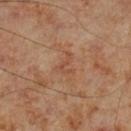| field | value |
|---|---|
| patient | male, in their 70s |
| tile lighting | cross-polarized illumination |
| image | 15 mm crop, total-body photography |
| lesion size | ≈2.5 mm |
| location | the left lower leg |
| automated lesion analysis | an average lesion color of about L≈47 a*≈22 b*≈31 (CIELAB), roughly 6 lightness units darker than nearby skin, and a normalized border contrast of about 4.5; a border-irregularity index near 8.5/10, a within-lesion color-variation index near 0/10, and peripheral color asymmetry of about 0; lesion-presence confidence of about 100/100 |